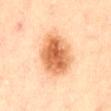follow-up = total-body-photography surveillance lesion; no biopsy | image source = ~15 mm crop, total-body skin-cancer survey | location = the abdomen | tile lighting = cross-polarized | subject = male, about 85 years old or older | size = ≈6 mm.